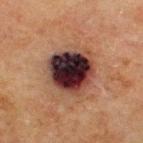Impression:
Part of a total-body skin-imaging series; this lesion was reviewed on a skin check and was not flagged for biopsy.
Context:
A 15 mm crop from a total-body photograph taken for skin-cancer surveillance. On the upper back. Automated image analysis of the tile measured an area of roughly 22 mm², a shape eccentricity near 0.3, and a symmetry-axis asymmetry near 0.15. The analysis additionally found internal color variation of about 10 on a 0–10 scale and radial color variation of about 3.5. A male subject roughly 70 years of age. Measured at roughly 5.5 mm in maximum diameter. The tile uses cross-polarized illumination.